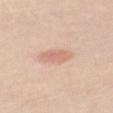Recorded during total-body skin imaging; not selected for excision or biopsy. Cropped from a whole-body photographic skin survey; the tile spans about 15 mm. The lesion is on the back. A female subject, aged 48 to 52. The tile uses white-light illumination. About 3 mm across.